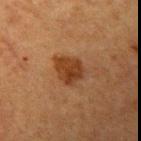Case summary:
- workup · catalogued during a skin exam; not biopsied
- image · 15 mm crop, total-body photography
- anatomic site · the arm
- patient · male, aged approximately 50